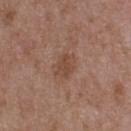| field | value |
|---|---|
| notes | no biopsy performed (imaged during a skin exam) |
| patient | male, in their 50s |
| automated lesion analysis | an area of roughly 5.5 mm², an eccentricity of roughly 0.65, and two-axis asymmetry of about 0.2; a nevus-likeness score of about 0/100 |
| site | the upper back |
| image source | total-body-photography crop, ~15 mm field of view |